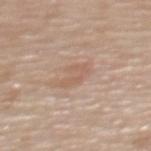Clinical impression: Imaged during a routine full-body skin examination; the lesion was not biopsied and no histopathology is available. Context: From the upper back. The lesion's longest dimension is about 3 mm. A female subject roughly 65 years of age. The lesion-visualizer software estimated a lesion area of about 5 mm², an outline eccentricity of about 0.75 (0 = round, 1 = elongated), and a shape-asymmetry score of about 0.35 (0 = symmetric). And it measured a border-irregularity index near 4.5/10, a color-variation rating of about 1/10, and radial color variation of about 0.5. And it measured a nevus-likeness score of about 10/100 and lesion-presence confidence of about 100/100. A lesion tile, about 15 mm wide, cut from a 3D total-body photograph.